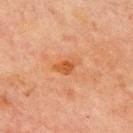Case summary:
– biopsy status — no biopsy performed (imaged during a skin exam)
– illumination — cross-polarized illumination
– patient — male, aged 68–72
– diameter — about 3 mm
– image — ~15 mm crop, total-body skin-cancer survey
– site — the chest